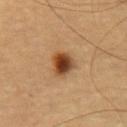The lesion was tiled from a total-body skin photograph and was not biopsied. The lesion's longest dimension is about 3 mm. The total-body-photography lesion software estimated an area of roughly 6.5 mm², an outline eccentricity of about 0.55 (0 = round, 1 = elongated), and a symmetry-axis asymmetry near 0.25. The analysis additionally found a nevus-likeness score of about 100/100 and lesion-presence confidence of about 100/100. Imaged with cross-polarized lighting. From the upper back. A male patient, aged 63–67. A 15 mm crop from a total-body photograph taken for skin-cancer surveillance.